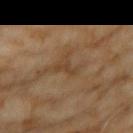  biopsy_status: not biopsied; imaged during a skin examination
  patient:
    sex: female
    age_approx: 60
  automated_metrics:
    area_mm2_approx: 4.5
    eccentricity: 0.8
    shape_asymmetry: 0.65
    cielab_L: 38
    cielab_a: 15
    cielab_b: 29
    vs_skin_contrast_norm: 5.5
    nevus_likeness_0_100: 0
    lesion_detection_confidence_0_100: 55
  image:
    source: total-body photography crop
    field_of_view_mm: 15
  lighting: cross-polarized
  lesion_size:
    long_diameter_mm_approx: 3.5
  site: left upper arm